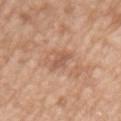Acquisition and patient details: Cropped from a total-body skin-imaging series; the visible field is about 15 mm. This is a white-light tile. The lesion is on the upper back. A male patient approximately 50 years of age. Approximately 3 mm at its widest. Automated tile analysis of the lesion measured an average lesion color of about L≈55 a*≈22 b*≈31 (CIELAB), a lesion–skin lightness drop of about 8, and a lesion-to-skin contrast of about 5.5 (normalized; higher = more distinct). And it measured border irregularity of about 4 on a 0–10 scale, a color-variation rating of about 0/10, and peripheral color asymmetry of about 0. It also reported a nevus-likeness score of about 0/100 and a lesion-detection confidence of about 100/100.